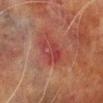Findings:
* workup — no biopsy performed (imaged during a skin exam)
* lesion size — ~3 mm (longest diameter)
* location — the right lower leg
* automated lesion analysis — an area of roughly 6.5 mm² and a shape eccentricity near 0.7; a mean CIELAB color near L≈35 a*≈28 b*≈23 and a normalized border contrast of about 5.5; border irregularity of about 4 on a 0–10 scale, internal color variation of about 5 on a 0–10 scale, and a peripheral color-asymmetry measure near 1.5; a classifier nevus-likeness of about 0/100 and a detector confidence of about 100 out of 100 that the crop contains a lesion
* patient — male, aged 68 to 72
* illumination — cross-polarized
* image source — 15 mm crop, total-body photography